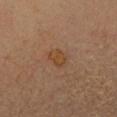• notes — imaged on a skin check; not biopsied
• lesion size — about 2.5 mm
• subject — female, aged approximately 35
• body site — the head or neck
• imaging modality — ~15 mm tile from a whole-body skin photo